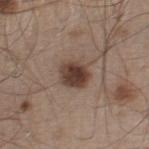The lesion was tiled from a total-body skin photograph and was not biopsied. A 15 mm close-up extracted from a 3D total-body photography capture. From the right thigh. Approximately 3.5 mm at its widest. A male subject aged 58–62. Captured under white-light illumination.